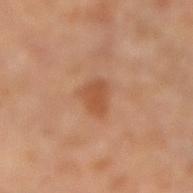Assessment:
Part of a total-body skin-imaging series; this lesion was reviewed on a skin check and was not flagged for biopsy.
Context:
Captured under cross-polarized illumination. A female subject aged 68–72. The lesion is on the right lower leg. A 15 mm close-up tile from a total-body photography series done for melanoma screening.